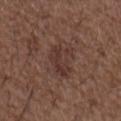follow-up: catalogued during a skin exam; not biopsied | subject: male, approximately 50 years of age | body site: the left upper arm | image: ~15 mm crop, total-body skin-cancer survey | diameter: about 4 mm.